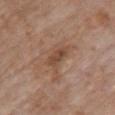biopsy_status: not biopsied; imaged during a skin examination
patient:
  sex: female
  age_approx: 85+
automated_metrics:
  border_irregularity_0_10: 3.5
  color_variation_0_10: 2.0
  peripheral_color_asymmetry: 0.5
  nevus_likeness_0_100: 0
  lesion_detection_confidence_0_100: 100
site: chest
lesion_size:
  long_diameter_mm_approx: 3.0
image:
  source: total-body photography crop
  field_of_view_mm: 15